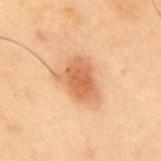Clinical impression:
The lesion was tiled from a total-body skin photograph and was not biopsied.
Acquisition and patient details:
The subject is a male roughly 55 years of age. A region of skin cropped from a whole-body photographic capture, roughly 15 mm wide. The lesion is located on the mid back. The recorded lesion diameter is about 4.5 mm. This is a cross-polarized tile.